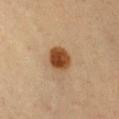Assessment:
Imaged during a routine full-body skin examination; the lesion was not biopsied and no histopathology is available.
Clinical summary:
The lesion is located on the chest. Cropped from a total-body skin-imaging series; the visible field is about 15 mm. Approximately 3 mm at its widest. A female subject, about 35 years old. Captured under cross-polarized illumination.